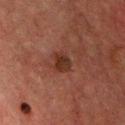Cropped from a whole-body photographic skin survey; the tile spans about 15 mm. The lesion is on the upper back. The recorded lesion diameter is about 2.5 mm. Imaged with cross-polarized lighting. The lesion-visualizer software estimated a lesion area of about 4.5 mm² and an eccentricity of roughly 0.6. The analysis additionally found a mean CIELAB color near L≈25 a*≈19 b*≈23. And it measured border irregularity of about 2.5 on a 0–10 scale, a within-lesion color-variation index near 2.5/10, and a peripheral color-asymmetry measure near 1. It also reported a classifier nevus-likeness of about 35/100 and lesion-presence confidence of about 100/100. A male patient, in their mid-60s.